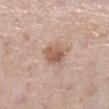Q: Was this lesion biopsied?
A: total-body-photography surveillance lesion; no biopsy
Q: What lighting was used for the tile?
A: white-light illumination
Q: How large is the lesion?
A: ≈3 mm
Q: Where on the body is the lesion?
A: the right lower leg
Q: Who is the patient?
A: female, in their mid-60s
Q: What is the imaging modality?
A: 15 mm crop, total-body photography